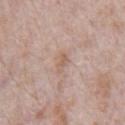notes: catalogued during a skin exam; not biopsied | illumination: white-light illumination | automated lesion analysis: an outline eccentricity of about 0.85 (0 = round, 1 = elongated) and a shape-asymmetry score of about 0.3 (0 = symmetric); border irregularity of about 3 on a 0–10 scale, a color-variation rating of about 0.5/10, and a peripheral color-asymmetry measure near 0; an automated nevus-likeness rating near 0 out of 100 and a detector confidence of about 100 out of 100 that the crop contains a lesion | diameter: ≈2.5 mm | subject: male, aged 68–72 | image source: ~15 mm tile from a whole-body skin photo | anatomic site: the chest.